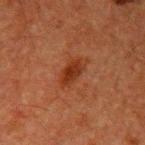Notes:
- notes · no biopsy performed (imaged during a skin exam)
- lesion size · about 3.5 mm
- subject · male, aged 58 to 62
- anatomic site · the arm
- tile lighting · cross-polarized
- automated lesion analysis · an area of roughly 5 mm² and a symmetry-axis asymmetry near 0.2; a border-irregularity rating of about 2/10, a within-lesion color-variation index near 2/10, and peripheral color asymmetry of about 0.5
- imaging modality · ~15 mm tile from a whole-body skin photo